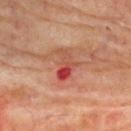This lesion was catalogued during total-body skin photography and was not selected for biopsy. The patient is a male in their mid-70s. Located on the upper back. A close-up tile cropped from a whole-body skin photograph, about 15 mm across. The tile uses cross-polarized illumination. The recorded lesion diameter is about 3.5 mm. Automated image analysis of the tile measured an area of roughly 6.5 mm² and two-axis asymmetry of about 0.45. It also reported a mean CIELAB color near L≈42 a*≈28 b*≈27, about 9 CIELAB-L* units darker than the surrounding skin, and a lesion-to-skin contrast of about 7.5 (normalized; higher = more distinct). It also reported a border-irregularity rating of about 5/10, internal color variation of about 10 on a 0–10 scale, and peripheral color asymmetry of about 6.